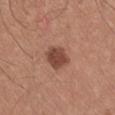Q: Was a biopsy performed?
A: catalogued during a skin exam; not biopsied
Q: What did automated image analysis measure?
A: an average lesion color of about L≈46 a*≈23 b*≈27 (CIELAB), a lesion–skin lightness drop of about 12, and a normalized border contrast of about 9; a border-irregularity index near 2/10, a within-lesion color-variation index near 2.5/10, and peripheral color asymmetry of about 1; a classifier nevus-likeness of about 80/100 and lesion-presence confidence of about 100/100
Q: Lesion location?
A: the left upper arm
Q: Patient demographics?
A: male, in their mid- to late 20s
Q: How was this image acquired?
A: ~15 mm tile from a whole-body skin photo
Q: How was the tile lit?
A: white-light illumination